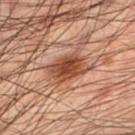Clinical impression:
Captured during whole-body skin photography for melanoma surveillance; the lesion was not biopsied.
Clinical summary:
The tile uses cross-polarized illumination. A male patient, about 50 years old. The lesion's longest dimension is about 5 mm. The lesion is located on the left lower leg. Automated tile analysis of the lesion measured about 11 CIELAB-L* units darker than the surrounding skin and a normalized lesion–skin contrast near 10. A 15 mm close-up extracted from a 3D total-body photography capture.